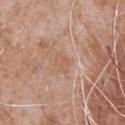Clinical impression:
Part of a total-body skin-imaging series; this lesion was reviewed on a skin check and was not flagged for biopsy.